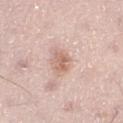biopsy_status: not biopsied; imaged during a skin examination
lesion_size:
  long_diameter_mm_approx: 3.0
site: left thigh
patient:
  sex: male
  age_approx: 55
lighting: white-light
image:
  source: total-body photography crop
  field_of_view_mm: 15